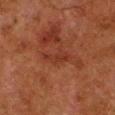follow-up = total-body-photography surveillance lesion; no biopsy
tile lighting = cross-polarized
automated lesion analysis = a footprint of about 4.5 mm²; a lesion color around L≈27 a*≈23 b*≈26 in CIELAB, a lesion–skin lightness drop of about 5, and a normalized lesion–skin contrast near 5.5; border irregularity of about 4.5 on a 0–10 scale, a color-variation rating of about 1/10, and peripheral color asymmetry of about 0.5
subject = male, approximately 80 years of age
body site = the left lower leg
image = total-body-photography crop, ~15 mm field of view
size = about 4 mm